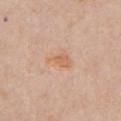Captured during whole-body skin photography for melanoma surveillance; the lesion was not biopsied. From the chest. The lesion-visualizer software estimated an area of roughly 4.5 mm² and an eccentricity of roughly 0.8. The software also gave internal color variation of about 1.5 on a 0–10 scale and radial color variation of about 0.5. The analysis additionally found a nevus-likeness score of about 5/100. Cropped from a total-body skin-imaging series; the visible field is about 15 mm. Approximately 3.5 mm at its widest. A female patient, about 55 years old.